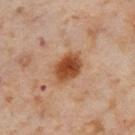notes: no biopsy performed (imaged during a skin exam) | site: the leg | lighting: cross-polarized | patient: female, aged 53 to 57 | image: ~15 mm tile from a whole-body skin photo.